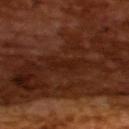The lesion was tiled from a total-body skin photograph and was not biopsied. A region of skin cropped from a whole-body photographic capture, roughly 15 mm wide. A male subject aged around 65.